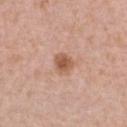Part of a total-body skin-imaging series; this lesion was reviewed on a skin check and was not flagged for biopsy. The patient is a female approximately 20 years of age. Approximately 2.5 mm at its widest. Captured under white-light illumination. A region of skin cropped from a whole-body photographic capture, roughly 15 mm wide. From the chest.